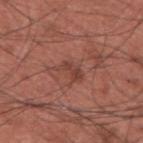{
  "biopsy_status": "not biopsied; imaged during a skin examination",
  "site": "upper back",
  "lighting": "white-light",
  "lesion_size": {
    "long_diameter_mm_approx": 3.5
  },
  "image": {
    "source": "total-body photography crop",
    "field_of_view_mm": 15
  },
  "patient": {
    "sex": "male",
    "age_approx": 35
  }
}